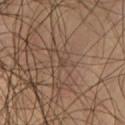Notes:
* workup · total-body-photography surveillance lesion; no biopsy
* illumination · cross-polarized illumination
* image · ~15 mm tile from a whole-body skin photo
* subject · male, aged 38 to 42
* lesion diameter · ~1 mm (longest diameter)
* site · the left thigh
* TBP lesion metrics · a border-irregularity rating of about 2.5/10 and peripheral color asymmetry of about 0; a detector confidence of about 0 out of 100 that the crop contains a lesion